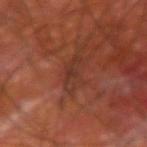{"biopsy_status": "not biopsied; imaged during a skin examination", "site": "right upper arm", "automated_metrics": {"eccentricity": 0.95, "shape_asymmetry": 0.25, "vs_skin_contrast_norm": 6.0, "border_irregularity_0_10": 3.5, "color_variation_0_10": 2.5, "peripheral_color_asymmetry": 1.0, "nevus_likeness_0_100": 0}, "lighting": "cross-polarized", "patient": {"sex": "male", "age_approx": 60}, "image": {"source": "total-body photography crop", "field_of_view_mm": 15}}